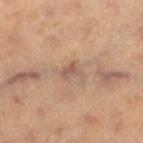The lesion was photographed on a routine skin check and not biopsied; there is no pathology result.
A female patient, aged 48 to 52.
On the left thigh.
A 15 mm close-up extracted from a 3D total-body photography capture.